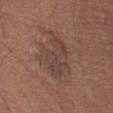The lesion was photographed on a routine skin check and not biopsied; there is no pathology result. A male subject aged 58 to 62. On the left thigh. The recorded lesion diameter is about 6 mm. Captured under white-light illumination. A lesion tile, about 15 mm wide, cut from a 3D total-body photograph.